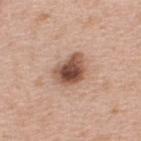patient=male, about 40 years old; location=the upper back; tile lighting=white-light illumination; TBP lesion metrics=a lesion–skin lightness drop of about 17; acquisition=~15 mm tile from a whole-body skin photo.